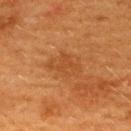Assessment:
Imaged during a routine full-body skin examination; the lesion was not biopsied and no histopathology is available.
Background:
The subject is a female aged 38 to 42. The lesion's longest dimension is about 4 mm. This image is a 15 mm lesion crop taken from a total-body photograph. From the upper back.